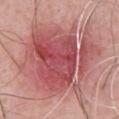Impression:
Recorded during total-body skin imaging; not selected for excision or biopsy.
Image and clinical context:
The subject is a male aged approximately 45. Imaged with white-light lighting. Approximately 12 mm at its widest. Cropped from a total-body skin-imaging series; the visible field is about 15 mm. The lesion is on the back.